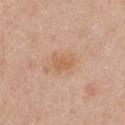biopsy status: imaged on a skin check; not biopsied
imaging modality: 15 mm crop, total-body photography
subject: female, approximately 40 years of age
lesion diameter: ≈2.5 mm
automated metrics: a mean CIELAB color near L≈61 a*≈21 b*≈34, a lesion–skin lightness drop of about 7, and a lesion-to-skin contrast of about 6 (normalized; higher = more distinct); a border-irregularity rating of about 2.5/10 and peripheral color asymmetry of about 0.5; a classifier nevus-likeness of about 5/100 and lesion-presence confidence of about 100/100
illumination: white-light
body site: the front of the torso